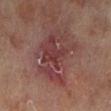Imaged during a routine full-body skin examination; the lesion was not biopsied and no histopathology is available. A female patient, in their 80s. This image is a 15 mm lesion crop taken from a total-body photograph. Located on the left lower leg. Measured at roughly 7 mm in maximum diameter.